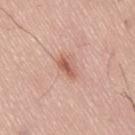biopsy_status: not biopsied; imaged during a skin examination
image:
  source: total-body photography crop
  field_of_view_mm: 15
automated_metrics:
  eccentricity: 0.85
  shape_asymmetry: 0.25
  nevus_likeness_0_100: 70
  lesion_detection_confidence_0_100: 100
lighting: white-light
patient:
  sex: male
  age_approx: 65
lesion_size:
  long_diameter_mm_approx: 3.0
site: lower back